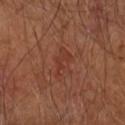biopsy status = total-body-photography surveillance lesion; no biopsy
size = ≈3.5 mm
body site = the right forearm
tile lighting = cross-polarized illumination
subject = male, aged 63–67
image-analysis metrics = a lesion–skin lightness drop of about 5
acquisition = total-body-photography crop, ~15 mm field of view